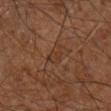size — ≈3 mm | anatomic site — the leg | image-analysis metrics — an outline eccentricity of about 0.75 (0 = round, 1 = elongated) and a symmetry-axis asymmetry near 0.3; a border-irregularity rating of about 3/10, internal color variation of about 1.5 on a 0–10 scale, and a peripheral color-asymmetry measure near 0.5 | imaging modality — ~15 mm crop, total-body skin-cancer survey | illumination — cross-polarized illumination | patient — aged approximately 65.